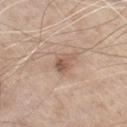Assessment: No biopsy was performed on this lesion — it was imaged during a full skin examination and was not determined to be concerning. Clinical summary: From the chest. Approximately 2.5 mm at its widest. Automated image analysis of the tile measured border irregularity of about 3 on a 0–10 scale and a color-variation rating of about 4.5/10. A male subject in their mid-60s. A region of skin cropped from a whole-body photographic capture, roughly 15 mm wide. The tile uses white-light illumination.